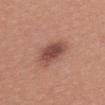Part of a total-body skin-imaging series; this lesion was reviewed on a skin check and was not flagged for biopsy. Longest diameter approximately 5 mm. A close-up tile cropped from a whole-body skin photograph, about 15 mm across. Automated tile analysis of the lesion measured a footprint of about 9.5 mm², an outline eccentricity of about 0.9 (0 = round, 1 = elongated), and a symmetry-axis asymmetry near 0.2. The analysis additionally found a border-irregularity rating of about 2.5/10, a within-lesion color-variation index near 5/10, and radial color variation of about 1.5. The software also gave a nevus-likeness score of about 60/100 and a lesion-detection confidence of about 100/100. A female patient roughly 20 years of age. The lesion is located on the upper back.